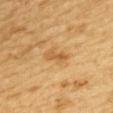Impression:
Part of a total-body skin-imaging series; this lesion was reviewed on a skin check and was not flagged for biopsy.
Context:
A lesion tile, about 15 mm wide, cut from a 3D total-body photograph. On the mid back. Automated image analysis of the tile measured a shape eccentricity near 0.95 and a shape-asymmetry score of about 0.3 (0 = symmetric). The analysis additionally found a lesion color around L≈59 a*≈21 b*≈46 in CIELAB, roughly 9 lightness units darker than nearby skin, and a normalized lesion–skin contrast near 6.5. The software also gave internal color variation of about 0 on a 0–10 scale. The software also gave a classifier nevus-likeness of about 5/100 and a detector confidence of about 100 out of 100 that the crop contains a lesion. The lesion's longest dimension is about 3.5 mm. The patient is a female roughly 55 years of age.